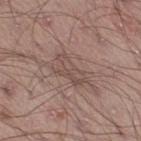Captured during whole-body skin photography for melanoma surveillance; the lesion was not biopsied.
A male patient approximately 20 years of age.
On the leg.
Automated image analysis of the tile measured a lesion area of about 6.5 mm², an eccentricity of roughly 0.85, and a symmetry-axis asymmetry near 0.5. The analysis additionally found a border-irregularity index near 6.5/10 and a peripheral color-asymmetry measure near 1.
A roughly 15 mm field-of-view crop from a total-body skin photograph.